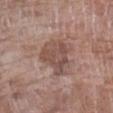Captured during whole-body skin photography for melanoma surveillance; the lesion was not biopsied.
A female patient aged approximately 70.
Automated tile analysis of the lesion measured about 9 CIELAB-L* units darker than the surrounding skin and a lesion-to-skin contrast of about 7 (normalized; higher = more distinct). The analysis additionally found a color-variation rating of about 4.5/10 and a peripheral color-asymmetry measure near 1.5.
The recorded lesion diameter is about 5 mm.
The lesion is located on the left forearm.
This is a white-light tile.
A close-up tile cropped from a whole-body skin photograph, about 15 mm across.